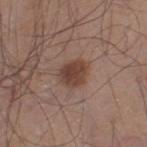{
  "biopsy_status": "not biopsied; imaged during a skin examination",
  "image": {
    "source": "total-body photography crop",
    "field_of_view_mm": 15
  },
  "site": "left thigh",
  "patient": {
    "sex": "male",
    "age_approx": 45
  },
  "lighting": "white-light"
}